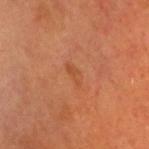Assessment: No biopsy was performed on this lesion — it was imaged during a full skin examination and was not determined to be concerning. Image and clinical context: The lesion is located on the head or neck. Captured under cross-polarized illumination. A 15 mm close-up extracted from a 3D total-body photography capture. A male patient, approximately 60 years of age. Measured at roughly 3 mm in maximum diameter.